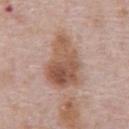Clinical impression: Part of a total-body skin-imaging series; this lesion was reviewed on a skin check and was not flagged for biopsy. Background: Located on the chest. The patient is a male aged around 70. This is a white-light tile. The lesion-visualizer software estimated a footprint of about 23 mm² and an outline eccentricity of about 0.75 (0 = round, 1 = elongated). The software also gave a border-irregularity index near 4/10 and a within-lesion color-variation index near 6.5/10. Cropped from a total-body skin-imaging series; the visible field is about 15 mm. The recorded lesion diameter is about 7 mm.